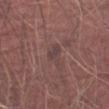{"biopsy_status": "not biopsied; imaged during a skin examination", "image": {"source": "total-body photography crop", "field_of_view_mm": 15}, "lighting": "white-light", "patient": {"sex": "male", "age_approx": 75}, "lesion_size": {"long_diameter_mm_approx": 3.0}, "site": "arm", "automated_metrics": {"area_mm2_approx": 4.0, "cielab_L": 41, "cielab_a": 16, "cielab_b": 17, "vs_skin_darker_L": 6.0, "vs_skin_contrast_norm": 6.0, "border_irregularity_0_10": 4.5, "color_variation_0_10": 2.5, "peripheral_color_asymmetry": 0.5, "nevus_likeness_0_100": 0, "lesion_detection_confidence_0_100": 80}}